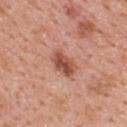Q: Was this lesion biopsied?
A: total-body-photography surveillance lesion; no biopsy
Q: What is the imaging modality?
A: total-body-photography crop, ~15 mm field of view
Q: Lesion location?
A: the upper back
Q: What is the lesion's diameter?
A: ≈3.5 mm
Q: Who is the patient?
A: male, aged around 60